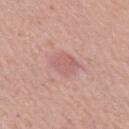notes: catalogued during a skin exam; not biopsied
imaging modality: ~15 mm tile from a whole-body skin photo
subject: male, aged 68–72
anatomic site: the right upper arm